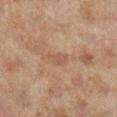Assessment:
Part of a total-body skin-imaging series; this lesion was reviewed on a skin check and was not flagged for biopsy.
Background:
Cropped from a total-body skin-imaging series; the visible field is about 15 mm. From the right lower leg. The lesion's longest dimension is about 2.5 mm. A female subject, in their 60s. This is a cross-polarized tile.